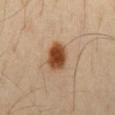Notes:
- notes · catalogued during a skin exam; not biopsied
- patient · male, aged 38 to 42
- body site · the chest
- acquisition · ~15 mm tile from a whole-body skin photo